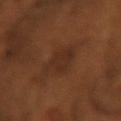| field | value |
|---|---|
| workup | imaged on a skin check; not biopsied |
| lesion diameter | about 4 mm |
| subject | male, in their mid-60s |
| body site | the right forearm |
| acquisition | total-body-photography crop, ~15 mm field of view |
| illumination | cross-polarized |
| TBP lesion metrics | an outline eccentricity of about 0.65 (0 = round, 1 = elongated) and a symmetry-axis asymmetry near 0.3; a border-irregularity index near 3.5/10, internal color variation of about 2 on a 0–10 scale, and radial color variation of about 1; a classifier nevus-likeness of about 0/100 |